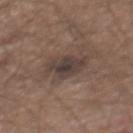{
  "lesion_size": {
    "long_diameter_mm_approx": 4.0
  },
  "lighting": "white-light",
  "site": "mid back",
  "patient": {
    "sex": "male",
    "age_approx": 65
  },
  "image": {
    "source": "total-body photography crop",
    "field_of_view_mm": 15
  }
}